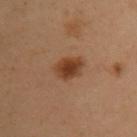Case summary:
• biopsy status: catalogued during a skin exam; not biopsied
• diameter: about 3.5 mm
• image source: 15 mm crop, total-body photography
• subject: female, aged 48 to 52
• location: the chest